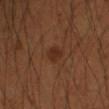No biopsy was performed on this lesion — it was imaged during a full skin examination and was not determined to be concerning.
The subject is a male aged approximately 40.
The total-body-photography lesion software estimated a lesion area of about 3.5 mm², an eccentricity of roughly 0.5, and two-axis asymmetry of about 0.2. And it measured a nevus-likeness score of about 95/100 and lesion-presence confidence of about 100/100.
The lesion's longest dimension is about 2 mm.
This is a cross-polarized tile.
Cropped from a total-body skin-imaging series; the visible field is about 15 mm.
Located on the left forearm.